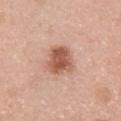Assessment: The lesion was tiled from a total-body skin photograph and was not biopsied. Acquisition and patient details: The total-body-photography lesion software estimated roughly 14 lightness units darker than nearby skin and a normalized border contrast of about 9. And it measured border irregularity of about 2.5 on a 0–10 scale, internal color variation of about 4.5 on a 0–10 scale, and radial color variation of about 1.5. Located on the chest. A female patient aged around 40. Imaged with white-light lighting. This image is a 15 mm lesion crop taken from a total-body photograph. Longest diameter approximately 3.5 mm.